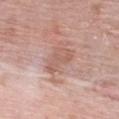notes — total-body-photography surveillance lesion; no biopsy | subject — female, aged around 70 | anatomic site — the chest | lighting — white-light | automated lesion analysis — internal color variation of about 2 on a 0–10 scale; a nevus-likeness score of about 0/100 and lesion-presence confidence of about 100/100 | diameter — ≈4 mm | imaging modality — ~15 mm tile from a whole-body skin photo.